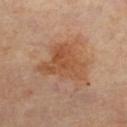{"patient": {"sex": "female", "age_approx": 60}, "lesion_size": {"long_diameter_mm_approx": 6.0}, "image": {"source": "total-body photography crop", "field_of_view_mm": 15}, "site": "right lower leg"}